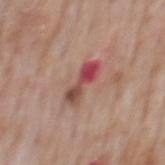Impression: Captured during whole-body skin photography for melanoma surveillance; the lesion was not biopsied. Image and clinical context: A male subject, approximately 75 years of age. The tile uses white-light illumination. A close-up tile cropped from a whole-body skin photograph, about 15 mm across. The lesion is located on the mid back. Approximately 4.5 mm at its widest.